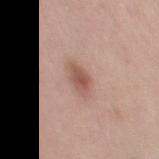  biopsy_status: not biopsied; imaged during a skin examination
  lesion_size:
    long_diameter_mm_approx: 3.5
  image:
    source: total-body photography crop
    field_of_view_mm: 15
  patient:
    sex: female
    age_approx: 50
  site: left thigh
  lighting: white-light